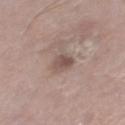Captured during whole-body skin photography for melanoma surveillance; the lesion was not biopsied. The patient is a male in their mid-70s. Located on the right thigh. A 15 mm close-up extracted from a 3D total-body photography capture. About 2.5 mm across. Imaged with white-light lighting.